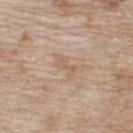biopsy status: imaged on a skin check; not biopsied
anatomic site: the back
patient: female, aged approximately 75
diameter: ~2.5 mm (longest diameter)
lighting: white-light
image: ~15 mm tile from a whole-body skin photo